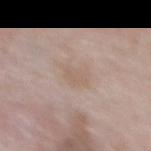Captured during whole-body skin photography for melanoma surveillance; the lesion was not biopsied.
Approximately 3.5 mm at its widest.
An algorithmic analysis of the crop reported a footprint of about 6 mm² and two-axis asymmetry of about 0.35. It also reported a border-irregularity index near 4/10, a within-lesion color-variation index near 1.5/10, and a peripheral color-asymmetry measure near 0.5. And it measured a detector confidence of about 100 out of 100 that the crop contains a lesion.
Imaged with white-light lighting.
From the upper back.
The patient is a female approximately 65 years of age.
A 15 mm close-up extracted from a 3D total-body photography capture.